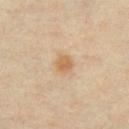Assessment: The lesion was photographed on a routine skin check and not biopsied; there is no pathology result. Acquisition and patient details: An algorithmic analysis of the crop reported a lesion area of about 4.5 mm², an outline eccentricity of about 0.3 (0 = round, 1 = elongated), and two-axis asymmetry of about 0.15. The software also gave an average lesion color of about L≈61 a*≈17 b*≈36 (CIELAB), roughly 8 lightness units darker than nearby skin, and a lesion-to-skin contrast of about 7 (normalized; higher = more distinct). It also reported a border-irregularity index near 1.5/10 and internal color variation of about 3 on a 0–10 scale. It also reported lesion-presence confidence of about 100/100. About 2.5 mm across. From the chest. A roughly 15 mm field-of-view crop from a total-body skin photograph. A female patient, in their mid-40s. This is a cross-polarized tile.